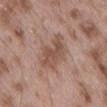<record>
<biopsy_status>not biopsied; imaged during a skin examination</biopsy_status>
<lighting>white-light</lighting>
<patient>
  <sex>male</sex>
  <age_approx>55</age_approx>
</patient>
<image>
  <source>total-body photography crop</source>
  <field_of_view_mm>15</field_of_view_mm>
</image>
<site>back</site>
<automated_metrics>
  <border_irregularity_0_10>5.5</border_irregularity_0_10>
  <color_variation_0_10>3.0</color_variation_0_10>
  <peripheral_color_asymmetry>1.0</peripheral_color_asymmetry>
</automated_metrics>
<lesion_size>
  <long_diameter_mm_approx>6.0</long_diameter_mm_approx>
</lesion_size>
</record>